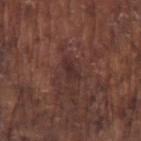Acquisition and patient details:
Cropped from a whole-body photographic skin survey; the tile spans about 15 mm. On the left upper arm. The lesion's longest dimension is about 3 mm. Automated image analysis of the tile measured an area of roughly 3.5 mm² and a shape eccentricity near 0.85. And it measured a mean CIELAB color near L≈29 a*≈19 b*≈19, a lesion–skin lightness drop of about 6, and a lesion-to-skin contrast of about 6.5 (normalized; higher = more distinct). The software also gave a lesion-detection confidence of about 90/100. Imaged with white-light lighting. A male patient aged around 75.